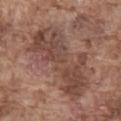The lesion was photographed on a routine skin check and not biopsied; there is no pathology result.
A 15 mm crop from a total-body photograph taken for skin-cancer surveillance.
The patient is a male aged 73 to 77.
Automated image analysis of the tile measured a lesion color around L≈45 a*≈19 b*≈25 in CIELAB and roughly 10 lightness units darker than nearby skin. The software also gave a within-lesion color-variation index near 4.5/10. It also reported a detector confidence of about 95 out of 100 that the crop contains a lesion.
Captured under white-light illumination.
The lesion is located on the abdomen.
About 10 mm across.